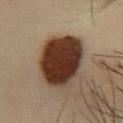- biopsy status: no biopsy performed (imaged during a skin exam)
- lesion size: ~6.5 mm (longest diameter)
- subject: male, in their mid-30s
- image source: 15 mm crop, total-body photography
- site: the leg
- image-analysis metrics: a lesion area of about 28 mm², an eccentricity of roughly 0.6, and a symmetry-axis asymmetry near 0.15
- illumination: cross-polarized illumination